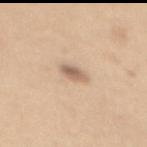Context:
Automated image analysis of the tile measured a shape eccentricity near 0.8. It also reported a mean CIELAB color near L≈62 a*≈17 b*≈29, roughly 13 lightness units darker than nearby skin, and a lesion-to-skin contrast of about 8 (normalized; higher = more distinct). On the mid back. A roughly 15 mm field-of-view crop from a total-body skin photograph. A female subject, about 30 years old.